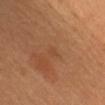Notes:
- notes · total-body-photography surveillance lesion; no biopsy
- diameter · ~1 mm (longest diameter)
- subject · female, approximately 30 years of age
- lighting · cross-polarized
- acquisition · 15 mm crop, total-body photography
- automated lesion analysis · an area of roughly 1 mm², an outline eccentricity of about 0.35 (0 = round, 1 = elongated), and two-axis asymmetry of about 0.3; a lesion color around L≈46 a*≈22 b*≈35 in CIELAB, roughly 4 lightness units darker than nearby skin, and a lesion-to-skin contrast of about 3.5 (normalized; higher = more distinct); border irregularity of about 2.5 on a 0–10 scale, internal color variation of about 0 on a 0–10 scale, and radial color variation of about 0
- location · the head or neck